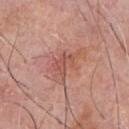Context:
The recorded lesion diameter is about 3.5 mm. A male patient, approximately 60 years of age. Captured under white-light illumination. A region of skin cropped from a whole-body photographic capture, roughly 15 mm wide. On the chest.